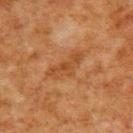Q: What is the lesion's diameter?
A: about 5 mm
Q: Patient demographics?
A: male, approximately 80 years of age
Q: Where on the body is the lesion?
A: the back
Q: How was the tile lit?
A: cross-polarized illumination
Q: How was this image acquired?
A: ~15 mm crop, total-body skin-cancer survey